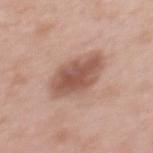Assessment: The lesion was photographed on a routine skin check and not biopsied; there is no pathology result.